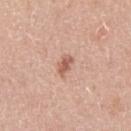<lesion>
<biopsy_status>not biopsied; imaged during a skin examination</biopsy_status>
<image>
  <source>total-body photography crop</source>
  <field_of_view_mm>15</field_of_view_mm>
</image>
<lighting>white-light</lighting>
<patient>
  <sex>male</sex>
  <age_approx>45</age_approx>
</patient>
<site>right upper arm</site>
</lesion>